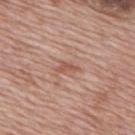Part of a total-body skin-imaging series; this lesion was reviewed on a skin check and was not flagged for biopsy. A close-up tile cropped from a whole-body skin photograph, about 15 mm across. The lesion is on the upper back. Captured under white-light illumination. The lesion's longest dimension is about 2.5 mm. Automated tile analysis of the lesion measured a border-irregularity index near 5/10, a color-variation rating of about 0/10, and radial color variation of about 0. The patient is a male aged 68–72.